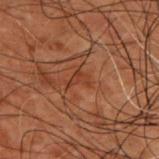Imaged during a routine full-body skin examination; the lesion was not biopsied and no histopathology is available.
The tile uses cross-polarized illumination.
Automated tile analysis of the lesion measured a footprint of about 2.5 mm², a shape eccentricity near 0.8, and a shape-asymmetry score of about 0.6 (0 = symmetric). It also reported a mean CIELAB color near L≈29 a*≈21 b*≈26, a lesion–skin lightness drop of about 5, and a normalized border contrast of about 6. The software also gave a nevus-likeness score of about 0/100 and a lesion-detection confidence of about 75/100.
Longest diameter approximately 2.5 mm.
Cropped from a total-body skin-imaging series; the visible field is about 15 mm.
The subject is a male approximately 50 years of age.
The lesion is on the upper back.